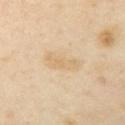The lesion is on the left upper arm. Automated tile analysis of the lesion measured a footprint of about 6 mm² and a shape-asymmetry score of about 0.3 (0 = symmetric). The analysis additionally found an average lesion color of about L≈72 a*≈13 b*≈38 (CIELAB), a lesion–skin lightness drop of about 6, and a normalized lesion–skin contrast near 4.5. The recorded lesion diameter is about 4 mm. A female patient aged approximately 40. Cropped from a total-body skin-imaging series; the visible field is about 15 mm. This is a cross-polarized tile.